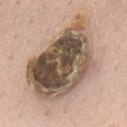{"biopsy_status": "not biopsied; imaged during a skin examination", "patient": {"sex": "male", "age_approx": 60}, "site": "mid back", "lighting": "white-light", "lesion_size": {"long_diameter_mm_approx": 10.5}, "image": {"source": "total-body photography crop", "field_of_view_mm": 15}}